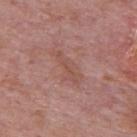The lesion was photographed on a routine skin check and not biopsied; there is no pathology result.
Approximately 4.5 mm at its widest.
Automated image analysis of the tile measured an area of roughly 5 mm² and a shape eccentricity near 0.95. And it measured a lesion color around L≈51 a*≈23 b*≈25 in CIELAB and a normalized lesion–skin contrast near 5. And it measured border irregularity of about 6 on a 0–10 scale and a within-lesion color-variation index near 0/10.
A 15 mm close-up extracted from a 3D total-body photography capture.
On the upper back.
The subject is a male aged approximately 75.